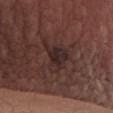The lesion was photographed on a routine skin check and not biopsied; there is no pathology result. The lesion is on the lower back. Imaged with white-light lighting. The subject is a male roughly 40 years of age. An algorithmic analysis of the crop reported an area of roughly 10 mm², an outline eccentricity of about 0.65 (0 = round, 1 = elongated), and a shape-asymmetry score of about 0.5 (0 = symmetric). It also reported a mean CIELAB color near L≈26 a*≈16 b*≈17 and roughly 7 lightness units darker than nearby skin. Cropped from a whole-body photographic skin survey; the tile spans about 15 mm.